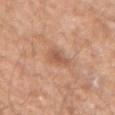Notes:
– notes — total-body-photography surveillance lesion; no biopsy
– imaging modality — ~15 mm tile from a whole-body skin photo
– automated metrics — an average lesion color of about L≈56 a*≈23 b*≈32 (CIELAB), a lesion–skin lightness drop of about 9, and a lesion-to-skin contrast of about 6 (normalized; higher = more distinct); a border-irregularity rating of about 3.5/10; a detector confidence of about 100 out of 100 that the crop contains a lesion
– site — the arm
– lighting — white-light
– patient — male, about 50 years old
– lesion size — about 2.5 mm